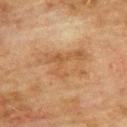biopsy status=total-body-photography surveillance lesion; no biopsy | size=~8 mm (longest diameter) | image source=~15 mm tile from a whole-body skin photo | subject=male, aged 73–77 | site=the upper back.